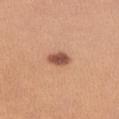The subject is a female in their mid- to late 20s.
The lesion is located on the right forearm.
A close-up tile cropped from a whole-body skin photograph, about 15 mm across.
The tile uses white-light illumination.